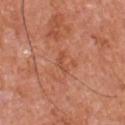Assessment:
This lesion was catalogued during total-body skin photography and was not selected for biopsy.
Clinical summary:
The tile uses white-light illumination. A male subject, aged approximately 55. The total-body-photography lesion software estimated a lesion area of about 2.5 mm² and a symmetry-axis asymmetry near 0.55. Located on the chest. A 15 mm close-up extracted from a 3D total-body photography capture.